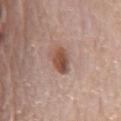Captured during whole-body skin photography for melanoma surveillance; the lesion was not biopsied.
About 3.5 mm across.
A male patient, approximately 80 years of age.
The lesion is on the chest.
This image is a 15 mm lesion crop taken from a total-body photograph.
Automated image analysis of the tile measured an area of roughly 5.5 mm², an eccentricity of roughly 0.75, and a shape-asymmetry score of about 0.25 (0 = symmetric). The analysis additionally found a border-irregularity index near 2.5/10, internal color variation of about 4.5 on a 0–10 scale, and radial color variation of about 1.5.
Captured under white-light illumination.